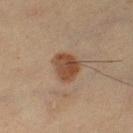biopsy status = total-body-photography surveillance lesion; no biopsy
site = the left thigh
lighting = cross-polarized
imaging modality = ~15 mm tile from a whole-body skin photo
size = ≈3.5 mm
patient = female, aged 53–57